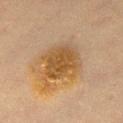{
  "biopsy_status": "not biopsied; imaged during a skin examination",
  "site": "right thigh",
  "lighting": "cross-polarized",
  "patient": {
    "sex": "female",
    "age_approx": 40
  },
  "image": {
    "source": "total-body photography crop",
    "field_of_view_mm": 15
  }
}